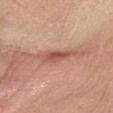Impression:
This lesion was catalogued during total-body skin photography and was not selected for biopsy.
Clinical summary:
Imaged with white-light lighting. A 15 mm close-up tile from a total-body photography series done for melanoma screening. From the left forearm. A male subject in their 70s. Longest diameter approximately 5 mm.